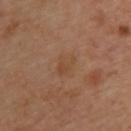The lesion was tiled from a total-body skin photograph and was not biopsied. From the upper back. An algorithmic analysis of the crop reported border irregularity of about 4.5 on a 0–10 scale, a color-variation rating of about 1.5/10, and a peripheral color-asymmetry measure near 0.5. The analysis additionally found lesion-presence confidence of about 100/100. A female subject approximately 40 years of age. A close-up tile cropped from a whole-body skin photograph, about 15 mm across. Approximately 3 mm at its widest.